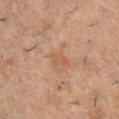A region of skin cropped from a whole-body photographic capture, roughly 15 mm wide. An algorithmic analysis of the crop reported an area of roughly 3 mm² and two-axis asymmetry of about 0.35. It also reported a lesion color around L≈42 a*≈16 b*≈26 in CIELAB, roughly 5 lightness units darker than nearby skin, and a normalized lesion–skin contrast near 5. The analysis additionally found a border-irregularity index near 3.5/10, internal color variation of about 0 on a 0–10 scale, and a peripheral color-asymmetry measure near 0. And it measured a nevus-likeness score of about 0/100. The subject is a male in their 50s. Located on the chest. Approximately 2.5 mm at its widest.